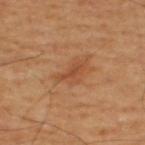biopsy_status: not biopsied; imaged during a skin examination
patient:
  sex: male
  age_approx: 55
lighting: cross-polarized
site: upper back
automated_metrics:
  area_mm2_approx: 2.5
  eccentricity: 0.9
  shape_asymmetry: 0.45
image:
  source: total-body photography crop
  field_of_view_mm: 15